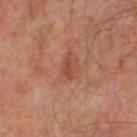Assessment: The lesion was photographed on a routine skin check and not biopsied; there is no pathology result. Image and clinical context: A roughly 15 mm field-of-view crop from a total-body skin photograph. On the left thigh. The lesion's longest dimension is about 2.5 mm. The total-body-photography lesion software estimated a lesion area of about 2.5 mm², an outline eccentricity of about 0.9 (0 = round, 1 = elongated), and a symmetry-axis asymmetry near 0.45. And it measured an average lesion color of about L≈44 a*≈26 b*≈30 (CIELAB), roughly 8 lightness units darker than nearby skin, and a normalized border contrast of about 6.5. And it measured a border-irregularity index near 4.5/10, a color-variation rating of about 0/10, and peripheral color asymmetry of about 0. It also reported a classifier nevus-likeness of about 0/100. The subject is a male in their mid- to late 60s. Captured under cross-polarized illumination.